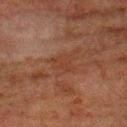Impression:
This lesion was catalogued during total-body skin photography and was not selected for biopsy.
Acquisition and patient details:
A male patient, about 80 years old. A roughly 15 mm field-of-view crop from a total-body skin photograph. The lesion is on the left upper arm.